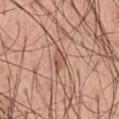The lesion is on the abdomen. The total-body-photography lesion software estimated an average lesion color of about L≈54 a*≈21 b*≈31 (CIELAB), a lesion–skin lightness drop of about 10, and a normalized lesion–skin contrast near 7.5. The analysis additionally found a classifier nevus-likeness of about 0/100 and a detector confidence of about 100 out of 100 that the crop contains a lesion. Measured at roughly 2.5 mm in maximum diameter. A close-up tile cropped from a whole-body skin photograph, about 15 mm across. Captured under white-light illumination. The subject is a male aged approximately 55.